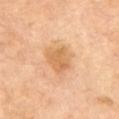Background: Located on the front of the torso. A lesion tile, about 15 mm wide, cut from a 3D total-body photograph. A male patient aged approximately 70. Automated image analysis of the tile measured a footprint of about 9 mm². The analysis additionally found roughly 9 lightness units darker than nearby skin and a normalized lesion–skin contrast near 6.5. Imaged with cross-polarized lighting. The recorded lesion diameter is about 4 mm.